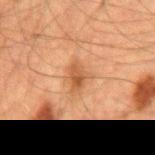Imaged during a routine full-body skin examination; the lesion was not biopsied and no histopathology is available. On the mid back. A male subject aged approximately 60. A lesion tile, about 15 mm wide, cut from a 3D total-body photograph.